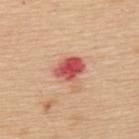follow-up: no biopsy performed (imaged during a skin exam).